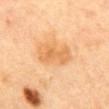The lesion was photographed on a routine skin check and not biopsied; there is no pathology result. A close-up tile cropped from a whole-body skin photograph, about 15 mm across. Captured under cross-polarized illumination. About 5.5 mm across. A female subject, in their 60s. The lesion is located on the mid back. An algorithmic analysis of the crop reported a border-irregularity rating of about 2.5/10, internal color variation of about 3.5 on a 0–10 scale, and peripheral color asymmetry of about 1.